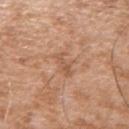Findings:
* follow-up: no biopsy performed (imaged during a skin exam)
* patient: male, in their mid-70s
* tile lighting: white-light
* automated metrics: a border-irregularity rating of about 5.5/10 and internal color variation of about 1.5 on a 0–10 scale; an automated nevus-likeness rating near 0 out of 100 and a detector confidence of about 100 out of 100 that the crop contains a lesion
* imaging modality: total-body-photography crop, ~15 mm field of view
* anatomic site: the arm
* lesion size: ~3.5 mm (longest diameter)